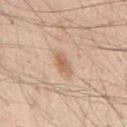workup: no biopsy performed (imaged during a skin exam)
site: the mid back
patient: male, about 45 years old
automated metrics: a shape eccentricity near 0.85 and a shape-asymmetry score of about 0.3 (0 = symmetric)
tile lighting: white-light illumination
size: ≈3 mm
imaging modality: ~15 mm crop, total-body skin-cancer survey